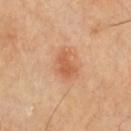Clinical impression: No biopsy was performed on this lesion — it was imaged during a full skin examination and was not determined to be concerning. Acquisition and patient details: An algorithmic analysis of the crop reported a nevus-likeness score of about 85/100. A 15 mm close-up extracted from a 3D total-body photography capture. This is a cross-polarized tile. Located on the chest.